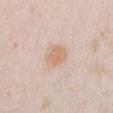Recorded during total-body skin imaging; not selected for excision or biopsy.
Located on the chest.
A close-up tile cropped from a whole-body skin photograph, about 15 mm across.
A female patient, aged 23 to 27.
This is a white-light tile.
The total-body-photography lesion software estimated a lesion-to-skin contrast of about 6.5 (normalized; higher = more distinct).
The recorded lesion diameter is about 3.5 mm.